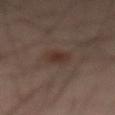Clinical impression: The lesion was tiled from a total-body skin photograph and was not biopsied. Image and clinical context: Cropped from a whole-body photographic skin survey; the tile spans about 15 mm. The lesion is located on the abdomen. The patient is a male approximately 65 years of age.